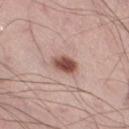biopsy status: no biopsy performed (imaged during a skin exam)
lesion diameter: ~3 mm (longest diameter)
anatomic site: the right thigh
subject: male, about 55 years old
acquisition: ~15 mm tile from a whole-body skin photo
image-analysis metrics: an outline eccentricity of about 0.6 (0 = round, 1 = elongated) and a shape-asymmetry score of about 0.2 (0 = symmetric); a nevus-likeness score of about 100/100 and lesion-presence confidence of about 100/100